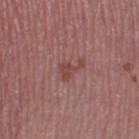biopsy status: catalogued during a skin exam; not biopsied | location: the left thigh | subject: female, approximately 45 years of age | diameter: about 3.5 mm | image: 15 mm crop, total-body photography | lighting: white-light illumination.